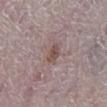Case summary:
* follow-up: imaged on a skin check; not biopsied
* imaging modality: ~15 mm tile from a whole-body skin photo
* subject: female, roughly 65 years of age
* lighting: white-light illumination
* size: ~2.5 mm (longest diameter)
* location: the leg
* image-analysis metrics: a border-irregularity rating of about 2.5/10 and a within-lesion color-variation index near 2.5/10